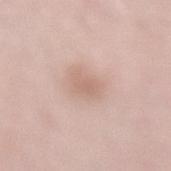The lesion was tiled from a total-body skin photograph and was not biopsied.
This image is a 15 mm lesion crop taken from a total-body photograph.
The recorded lesion diameter is about 2.5 mm.
The total-body-photography lesion software estimated a border-irregularity rating of about 3.5/10, internal color variation of about 1.5 on a 0–10 scale, and radial color variation of about 0.5. The analysis additionally found an automated nevus-likeness rating near 15 out of 100.
Captured under white-light illumination.
A male patient, about 55 years old.
Located on the lower back.